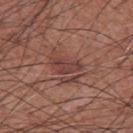Imaged during a routine full-body skin examination; the lesion was not biopsied and no histopathology is available. A close-up tile cropped from a whole-body skin photograph, about 15 mm across. On the chest. A male patient aged around 75. Measured at roughly 3.5 mm in maximum diameter. Captured under white-light illumination.